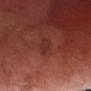Imaged during a routine full-body skin examination; the lesion was not biopsied and no histopathology is available.